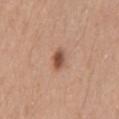{
  "biopsy_status": "not biopsied; imaged during a skin examination",
  "automated_metrics": {
    "area_mm2_approx": 4.0,
    "shape_asymmetry": 0.2,
    "color_variation_0_10": 4.5,
    "peripheral_color_asymmetry": 1.5
  },
  "lesion_size": {
    "long_diameter_mm_approx": 2.5
  },
  "patient": {
    "sex": "male",
    "age_approx": 40
  },
  "image": {
    "source": "total-body photography crop",
    "field_of_view_mm": 15
  },
  "site": "mid back"
}